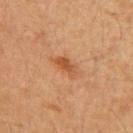<lesion>
  <biopsy_status>not biopsied; imaged during a skin examination</biopsy_status>
  <patient>
    <sex>female</sex>
    <age_approx>70</age_approx>
  </patient>
  <lighting>cross-polarized</lighting>
  <image>
    <source>total-body photography crop</source>
    <field_of_view_mm>15</field_of_view_mm>
  </image>
  <site>right upper arm</site>
  <lesion_size>
    <long_diameter_mm_approx>3.0</long_diameter_mm_approx>
  </lesion_size>
</lesion>